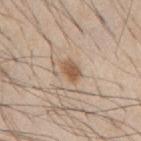notes: no biopsy performed (imaged during a skin exam) | lesion size: ≈3 mm | image: total-body-photography crop, ~15 mm field of view | automated lesion analysis: an automated nevus-likeness rating near 95 out of 100 and a lesion-detection confidence of about 100/100 | anatomic site: the mid back | subject: male, in their mid- to late 40s.